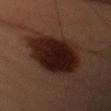Imaged during a routine full-body skin examination; the lesion was not biopsied and no histopathology is available. Located on the left upper arm. Measured at roughly 9 mm in maximum diameter. A lesion tile, about 15 mm wide, cut from a 3D total-body photograph. The tile uses cross-polarized illumination. A male subject, aged approximately 55.